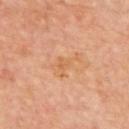Part of a total-body skin-imaging series; this lesion was reviewed on a skin check and was not flagged for biopsy. The lesion is located on the upper back. The tile uses cross-polarized illumination. The lesion's longest dimension is about 4 mm. A lesion tile, about 15 mm wide, cut from a 3D total-body photograph. Automated image analysis of the tile measured an eccentricity of roughly 0.85. A male patient aged approximately 60.